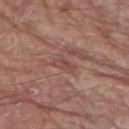* biopsy status — total-body-photography surveillance lesion; no biopsy
* patient — male, aged approximately 80
* image — total-body-photography crop, ~15 mm field of view
* location — the leg
* lesion diameter — about 3 mm